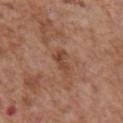{"biopsy_status": "not biopsied; imaged during a skin examination", "image": {"source": "total-body photography crop", "field_of_view_mm": 15}, "site": "chest", "patient": {"sex": "male", "age_approx": 65}}